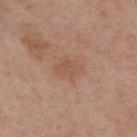Q: Was a biopsy performed?
A: no biopsy performed (imaged during a skin exam)
Q: How was the tile lit?
A: white-light
Q: What is the imaging modality?
A: total-body-photography crop, ~15 mm field of view
Q: Where on the body is the lesion?
A: the upper back
Q: Who is the patient?
A: male, approximately 55 years of age
Q: What did automated image analysis measure?
A: an eccentricity of roughly 0.65